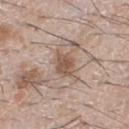workup = no biopsy performed (imaged during a skin exam) | image source = 15 mm crop, total-body photography | lesion diameter = about 3.5 mm | subject = male, aged 63–67 | anatomic site = the chest | illumination = white-light illumination.